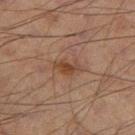The lesion was photographed on a routine skin check and not biopsied; there is no pathology result. The lesion-visualizer software estimated a lesion area of about 4.5 mm² and a shape eccentricity near 0.8. The analysis additionally found a border-irregularity index near 3.5/10, internal color variation of about 3 on a 0–10 scale, and radial color variation of about 1. It also reported a detector confidence of about 100 out of 100 that the crop contains a lesion. On the left thigh. A lesion tile, about 15 mm wide, cut from a 3D total-body photograph. A male subject in their 70s. This is a cross-polarized tile.